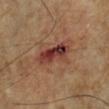• workup · catalogued during a skin exam; not biopsied
• automated lesion analysis · a mean CIELAB color near L≈35 a*≈24 b*≈25, about 13 CIELAB-L* units darker than the surrounding skin, and a normalized lesion–skin contrast near 11.5
• illumination · cross-polarized
• location · the leg
• image · 15 mm crop, total-body photography
• lesion size · ~5 mm (longest diameter)
• patient · male, roughly 65 years of age